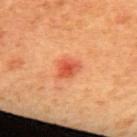{"biopsy_status": "not biopsied; imaged during a skin examination"}